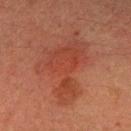{
  "lighting": "cross-polarized",
  "patient": {
    "sex": "female",
    "age_approx": 55
  },
  "site": "arm",
  "automated_metrics": {
    "area_mm2_approx": 25.0,
    "cielab_L": 35,
    "cielab_a": 24,
    "cielab_b": 27,
    "vs_skin_darker_L": 6.0,
    "vs_skin_contrast_norm": 5.5,
    "nevus_likeness_0_100": 15,
    "lesion_detection_confidence_0_100": 100
  },
  "image": {
    "source": "total-body photography crop",
    "field_of_view_mm": 15
  },
  "lesion_size": {
    "long_diameter_mm_approx": 8.5
  }
}